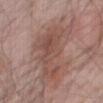Q: Lesion location?
A: the front of the torso
Q: What are the patient's age and sex?
A: male, aged 78–82
Q: What kind of image is this?
A: ~15 mm tile from a whole-body skin photo
Q: How large is the lesion?
A: about 10 mm
Q: Illumination type?
A: white-light illumination